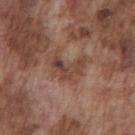anatomic site: the chest
lesion diameter: ≈4 mm
subject: male, roughly 75 years of age
lighting: white-light illumination
acquisition: 15 mm crop, total-body photography
TBP lesion metrics: a shape eccentricity near 0.8 and a shape-asymmetry score of about 0.5 (0 = symmetric); an average lesion color of about L≈43 a*≈19 b*≈26 (CIELAB), a lesion–skin lightness drop of about 9, and a lesion-to-skin contrast of about 7 (normalized; higher = more distinct); border irregularity of about 6.5 on a 0–10 scale and a peripheral color-asymmetry measure near 3; a nevus-likeness score of about 0/100 and a detector confidence of about 100 out of 100 that the crop contains a lesion